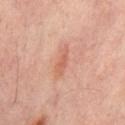This lesion was catalogued during total-body skin photography and was not selected for biopsy. On the lower back. Cropped from a whole-body photographic skin survey; the tile spans about 15 mm. The total-body-photography lesion software estimated an area of roughly 4 mm². It also reported a lesion color around L≈58 a*≈24 b*≈31 in CIELAB and a lesion–skin lightness drop of about 8. The software also gave a peripheral color-asymmetry measure near 0. It also reported a lesion-detection confidence of about 100/100. A male patient approximately 65 years of age. The recorded lesion diameter is about 3.5 mm.